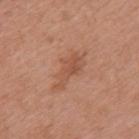Clinical impression:
Imaged during a routine full-body skin examination; the lesion was not biopsied and no histopathology is available.
Clinical summary:
A male subject, roughly 70 years of age. A 15 mm crop from a total-body photograph taken for skin-cancer surveillance. From the mid back.